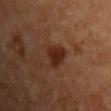Imaged during a routine full-body skin examination; the lesion was not biopsied and no histopathology is available.